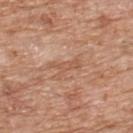Background:
This is a white-light tile. The lesion is on the upper back. A male patient, aged 78 to 82. Cropped from a whole-body photographic skin survey; the tile spans about 15 mm.